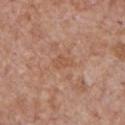Part of a total-body skin-imaging series; this lesion was reviewed on a skin check and was not flagged for biopsy. A male patient roughly 65 years of age. A 15 mm close-up extracted from a 3D total-body photography capture. On the front of the torso.